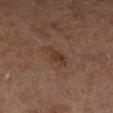Recorded during total-body skin imaging; not selected for excision or biopsy. A male patient in their 70s. Automated tile analysis of the lesion measured a lesion color around L≈27 a*≈15 b*≈22 in CIELAB, about 5 CIELAB-L* units darker than the surrounding skin, and a normalized border contrast of about 6.5. The lesion is on the right lower leg. A close-up tile cropped from a whole-body skin photograph, about 15 mm across. Captured under cross-polarized illumination. Approximately 3.5 mm at its widest.